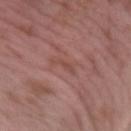* workup: total-body-photography surveillance lesion; no biopsy
* acquisition: total-body-photography crop, ~15 mm field of view
* illumination: white-light
* automated lesion analysis: a mean CIELAB color near L≈47 a*≈23 b*≈24, a lesion–skin lightness drop of about 6, and a lesion-to-skin contrast of about 5 (normalized; higher = more distinct); a border-irregularity rating of about 5/10, a within-lesion color-variation index near 0/10, and peripheral color asymmetry of about 0
* site: the left forearm
* lesion size: ≈2.5 mm
* patient: male, in their 40s